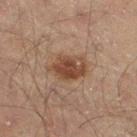This lesion was catalogued during total-body skin photography and was not selected for biopsy.
A roughly 15 mm field-of-view crop from a total-body skin photograph.
The tile uses cross-polarized illumination.
From the right thigh.
A male subject, aged approximately 60.
Measured at roughly 4.5 mm in maximum diameter.